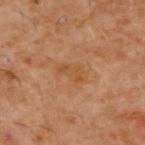biopsy status: imaged on a skin check; not biopsied | tile lighting: cross-polarized | lesion size: ~3 mm (longest diameter) | automated metrics: an area of roughly 5 mm², an outline eccentricity of about 0.85 (0 = round, 1 = elongated), and two-axis asymmetry of about 0.3; a lesion color around L≈47 a*≈21 b*≈36 in CIELAB and a lesion-to-skin contrast of about 5 (normalized; higher = more distinct); peripheral color asymmetry of about 0.5; a nevus-likeness score of about 0/100 | subject: male, aged 58–62 | anatomic site: the upper back | image: ~15 mm crop, total-body skin-cancer survey.